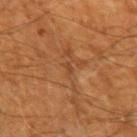This lesion was catalogued during total-body skin photography and was not selected for biopsy. Measured at roughly 1.5 mm in maximum diameter. This image is a 15 mm lesion crop taken from a total-body photograph. From the left upper arm. The patient is a male approximately 60 years of age.